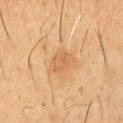Findings:
• follow-up — total-body-photography surveillance lesion; no biopsy
• location — the front of the torso
• lesion diameter — ~3 mm (longest diameter)
• automated metrics — a lesion color around L≈57 a*≈20 b*≈39 in CIELAB and roughly 6 lightness units darker than nearby skin; a border-irregularity index near 3/10 and a peripheral color-asymmetry measure near 1
• patient — male, aged 58–62
• lighting — cross-polarized illumination
• acquisition — ~15 mm tile from a whole-body skin photo